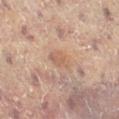Assessment: This lesion was catalogued during total-body skin photography and was not selected for biopsy. Background: The patient is a female approximately 80 years of age. Approximately 3 mm at its widest. On the leg. A close-up tile cropped from a whole-body skin photograph, about 15 mm across.